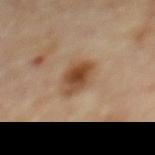The lesion was photographed on a routine skin check and not biopsied; there is no pathology result.
A region of skin cropped from a whole-body photographic capture, roughly 15 mm wide.
This is a cross-polarized tile.
A male patient, in their mid- to late 80s or older.
The lesion is on the back.
An algorithmic analysis of the crop reported an average lesion color of about L≈46 a*≈19 b*≈32 (CIELAB), a lesion–skin lightness drop of about 12, and a normalized lesion–skin contrast near 9.5. The analysis additionally found a border-irregularity index near 2.5/10, internal color variation of about 6 on a 0–10 scale, and radial color variation of about 1.5. The software also gave an automated nevus-likeness rating near 95 out of 100 and lesion-presence confidence of about 100/100.